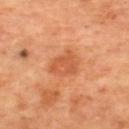follow-up=total-body-photography surveillance lesion; no biopsy | imaging modality=~15 mm crop, total-body skin-cancer survey | patient=female, about 45 years old | site=the upper back.